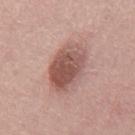Assessment: This lesion was catalogued during total-body skin photography and was not selected for biopsy. Background: The recorded lesion diameter is about 6 mm. On the mid back. The total-body-photography lesion software estimated an area of roughly 19 mm² and a shape eccentricity near 0.75. A 15 mm close-up tile from a total-body photography series done for melanoma screening. A male patient, aged approximately 45.